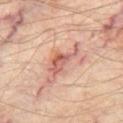Part of a total-body skin-imaging series; this lesion was reviewed on a skin check and was not flagged for biopsy. A patient in their mid-50s. A close-up tile cropped from a whole-body skin photograph, about 15 mm across. The lesion is located on the left thigh. Captured under cross-polarized illumination. Measured at roughly 4.5 mm in maximum diameter.